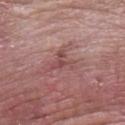Part of a total-body skin-imaging series; this lesion was reviewed on a skin check and was not flagged for biopsy. The lesion is on the left forearm. A 15 mm close-up tile from a total-body photography series done for melanoma screening. A male subject about 60 years old.